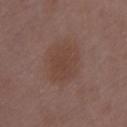workup = catalogued during a skin exam; not biopsied | lesion size = about 4 mm | anatomic site = the arm | image = ~15 mm tile from a whole-body skin photo | patient = female, aged 28–32.